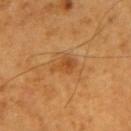No biopsy was performed on this lesion — it was imaged during a full skin examination and was not determined to be concerning.
A roughly 15 mm field-of-view crop from a total-body skin photograph.
A male subject, aged approximately 60.
On the left upper arm.